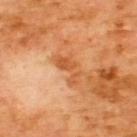biopsy status — imaged on a skin check; not biopsied
image — ~15 mm tile from a whole-body skin photo
TBP lesion metrics — a lesion area of about 7 mm² and an outline eccentricity of about 0.9 (0 = round, 1 = elongated); an average lesion color of about L≈55 a*≈28 b*≈42 (CIELAB), a lesion–skin lightness drop of about 10, and a lesion-to-skin contrast of about 7 (normalized; higher = more distinct)
subject — male, in their mid-60s
anatomic site — the upper back
tile lighting — cross-polarized